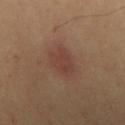biopsy status = total-body-photography surveillance lesion; no biopsy | acquisition = ~15 mm tile from a whole-body skin photo | automated lesion analysis = an eccentricity of roughly 0.85; an automated nevus-likeness rating near 85 out of 100 and a detector confidence of about 100 out of 100 that the crop contains a lesion | tile lighting = cross-polarized illumination | body site = the front of the torso | subject = male, roughly 65 years of age.